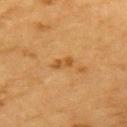  biopsy_status: not biopsied; imaged during a skin examination
  automated_metrics:
    area_mm2_approx: 3.5
    eccentricity: 0.9
    shape_asymmetry: 0.25
    cielab_L: 47
    cielab_a: 20
    cielab_b: 40
    nevus_likeness_0_100: 5
    lesion_detection_confidence_0_100: 100
  lesion_size:
    long_diameter_mm_approx: 3.0
  patient:
    sex: male
    age_approx: 85+
  site: upper back
  lighting: cross-polarized
  image:
    source: total-body photography crop
    field_of_view_mm: 15